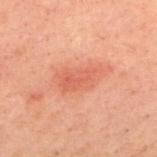biopsy_status: not biopsied; imaged during a skin examination
image:
  source: total-body photography crop
  field_of_view_mm: 15
site: upper back
patient:
  sex: male
  age_approx: 40
lesion_size:
  long_diameter_mm_approx: 5.5